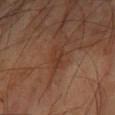Notes:
• follow-up — imaged on a skin check; not biopsied
• subject — male, aged 68–72
• tile lighting — cross-polarized illumination
• image source — ~15 mm tile from a whole-body skin photo
• site — the left forearm